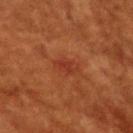Impression: The lesion was tiled from a total-body skin photograph and was not biopsied. Image and clinical context: Cropped from a total-body skin-imaging series; the visible field is about 15 mm. The lesion is located on the upper back. The subject is a female aged around 80.